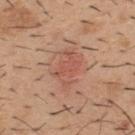No biopsy was performed on this lesion — it was imaged during a full skin examination and was not determined to be concerning. The total-body-photography lesion software estimated a lesion area of about 10 mm², an outline eccentricity of about 0.75 (0 = round, 1 = elongated), and a shape-asymmetry score of about 0.3 (0 = symmetric). And it measured an average lesion color of about L≈55 a*≈25 b*≈30 (CIELAB), a lesion–skin lightness drop of about 7, and a normalized border contrast of about 5. A 15 mm close-up tile from a total-body photography series done for melanoma screening. A male subject roughly 40 years of age. Located on the upper back. Captured under white-light illumination. The lesion's longest dimension is about 4.5 mm.